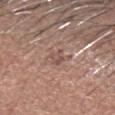Q: Automated lesion metrics?
A: a mean CIELAB color near L≈51 a*≈18 b*≈24, roughly 8 lightness units darker than nearby skin, and a normalized lesion–skin contrast near 5.5; a border-irregularity rating of about 4/10, a color-variation rating of about 2/10, and peripheral color asymmetry of about 1
Q: How large is the lesion?
A: ≈2.5 mm
Q: Where on the body is the lesion?
A: the head or neck
Q: What is the imaging modality?
A: ~15 mm tile from a whole-body skin photo
Q: How was the tile lit?
A: white-light
Q: What are the patient's age and sex?
A: male, in their mid-60s